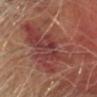Clinical impression:
Part of a total-body skin-imaging series; this lesion was reviewed on a skin check and was not flagged for biopsy.
Background:
Longest diameter approximately 12 mm. The subject is roughly 65 years of age. A 15 mm close-up tile from a total-body photography series done for melanoma screening. On the head or neck. Captured under cross-polarized illumination.